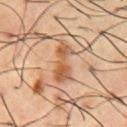Case summary:
– workup: total-body-photography surveillance lesion; no biopsy
– size: ~4.5 mm (longest diameter)
– image: ~15 mm tile from a whole-body skin photo
– lighting: cross-polarized illumination
– image-analysis metrics: an area of roughly 8 mm² and a shape-asymmetry score of about 0.4 (0 = symmetric); a classifier nevus-likeness of about 0/100
– body site: the chest
– patient: male, aged around 55